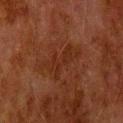Located on the head or neck.
Approximately 5 mm at its widest.
A male patient aged 78 to 82.
The total-body-photography lesion software estimated a nevus-likeness score of about 0/100.
A close-up tile cropped from a whole-body skin photograph, about 15 mm across.